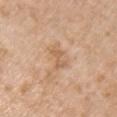Clinical impression: Part of a total-body skin-imaging series; this lesion was reviewed on a skin check and was not flagged for biopsy. Background: From the right upper arm. A 15 mm close-up extracted from a 3D total-body photography capture. A female subject, roughly 75 years of age. Approximately 2.5 mm at its widest. This is a white-light tile.